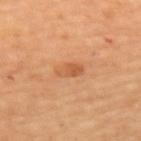Notes:
– body site · the upper back
– subject · female, approximately 70 years of age
– imaging modality · total-body-photography crop, ~15 mm field of view